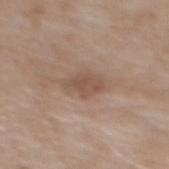Imaged during a routine full-body skin examination; the lesion was not biopsied and no histopathology is available.
A roughly 15 mm field-of-view crop from a total-body skin photograph.
From the mid back.
A female patient, aged 73–77.
This is a white-light tile.